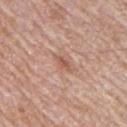Acquisition and patient details:
Imaged with white-light lighting. The lesion is on the arm. The patient is a male in their 70s. A 15 mm close-up extracted from a 3D total-body photography capture. An algorithmic analysis of the crop reported an average lesion color of about L≈56 a*≈22 b*≈29 (CIELAB) and a normalized border contrast of about 6.5. The analysis additionally found a classifier nevus-likeness of about 0/100 and a lesion-detection confidence of about 100/100. Approximately 2.5 mm at its widest.